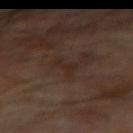No biopsy was performed on this lesion — it was imaged during a full skin examination and was not determined to be concerning. The lesion's longest dimension is about 3 mm. A male patient, roughly 70 years of age. Cropped from a whole-body photographic skin survey; the tile spans about 15 mm. Automated image analysis of the tile measured a shape-asymmetry score of about 0.6 (0 = symmetric). And it measured border irregularity of about 7 on a 0–10 scale, a color-variation rating of about 0/10, and radial color variation of about 0. The analysis additionally found a nevus-likeness score of about 0/100 and a lesion-detection confidence of about 95/100. The lesion is located on the right forearm.